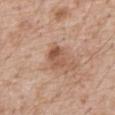  biopsy_status: not biopsied; imaged during a skin examination
  lesion_size:
    long_diameter_mm_approx: 3.0
  image:
    source: total-body photography crop
    field_of_view_mm: 15
  site: abdomen
  lighting: white-light
  patient:
    sex: male
    age_approx: 60